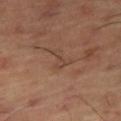This lesion was catalogued during total-body skin photography and was not selected for biopsy.
The lesion is on the right thigh.
A male patient approximately 65 years of age.
The total-body-photography lesion software estimated an automated nevus-likeness rating near 0 out of 100.
The tile uses cross-polarized illumination.
A lesion tile, about 15 mm wide, cut from a 3D total-body photograph.